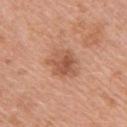workup: imaged on a skin check; not biopsied | subject: female, aged approximately 40 | tile lighting: white-light illumination | image-analysis metrics: a lesion area of about 9 mm² and two-axis asymmetry of about 0.3; a lesion color around L≈55 a*≈24 b*≈33 in CIELAB and a normalized lesion–skin contrast near 7; a border-irregularity rating of about 3.5/10 and a within-lesion color-variation index near 4/10 | body site: the right upper arm | lesion size: ≈4 mm | acquisition: ~15 mm tile from a whole-body skin photo.